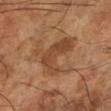follow-up=imaged on a skin check; not biopsied